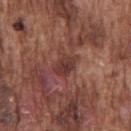Q: Was this lesion biopsied?
A: no biopsy performed (imaged during a skin exam)
Q: How was this image acquired?
A: total-body-photography crop, ~15 mm field of view
Q: What did automated image analysis measure?
A: a lesion area of about 6 mm² and a shape eccentricity near 0.75; a mean CIELAB color near L≈37 a*≈23 b*≈23, about 9 CIELAB-L* units darker than the surrounding skin, and a normalized lesion–skin contrast near 7.5; a border-irregularity index near 2.5/10, a color-variation rating of about 4/10, and a peripheral color-asymmetry measure near 1.5; a classifier nevus-likeness of about 0/100
Q: Lesion size?
A: ~3.5 mm (longest diameter)
Q: Patient demographics?
A: male, aged around 75
Q: How was the tile lit?
A: white-light illumination
Q: Lesion location?
A: the chest